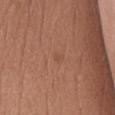Impression:
This lesion was catalogued during total-body skin photography and was not selected for biopsy.
Clinical summary:
This is a white-light tile. Automated tile analysis of the lesion measured a shape eccentricity near 0.6. The lesion is on the abdomen. A 15 mm close-up tile from a total-body photography series done for melanoma screening. The recorded lesion diameter is about 1 mm. A female subject, about 60 years old.